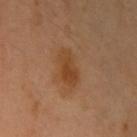The lesion was photographed on a routine skin check and not biopsied; there is no pathology result.
The patient is a male aged around 40.
Located on the left upper arm.
This image is a 15 mm lesion crop taken from a total-body photograph.
The tile uses cross-polarized illumination.